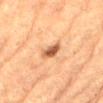{
  "biopsy_status": "not biopsied; imaged during a skin examination",
  "image": {
    "source": "total-body photography crop",
    "field_of_view_mm": 15
  },
  "patient": {
    "sex": "male",
    "age_approx": "85+"
  },
  "lighting": "cross-polarized",
  "automated_metrics": {
    "vs_skin_darker_L": 15.0,
    "peripheral_color_asymmetry": 1.0,
    "nevus_likeness_0_100": 70,
    "lesion_detection_confidence_0_100": 100
  },
  "site": "lower back"
}